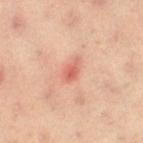Case summary:
• workup: no biopsy performed (imaged during a skin exam)
• imaging modality: 15 mm crop, total-body photography
• illumination: cross-polarized
• site: the right thigh
• patient: female, aged 38–42
• lesion size: about 3 mm